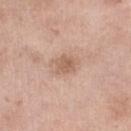biopsy status: no biopsy performed (imaged during a skin exam)
subject: female, aged around 55
imaging modality: ~15 mm tile from a whole-body skin photo
lesion size: ~2.5 mm (longest diameter)
body site: the leg